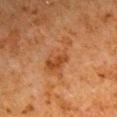Q: Was a biopsy performed?
A: imaged on a skin check; not biopsied
Q: What is the anatomic site?
A: the right upper arm
Q: How was this image acquired?
A: ~15 mm tile from a whole-body skin photo
Q: Patient demographics?
A: male, aged 78 to 82